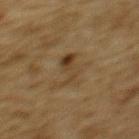Impression: Captured during whole-body skin photography for melanoma surveillance; the lesion was not biopsied. Context: The subject is a male aged approximately 85 or older. A 15 mm close-up extracted from a 3D total-body photography capture. Automated image analysis of the tile measured an average lesion color of about L≈34 a*≈12 b*≈28 (CIELAB), roughly 7 lightness units darker than nearby skin, and a lesion-to-skin contrast of about 6.5 (normalized; higher = more distinct). The lesion is on the upper back. Approximately 4 mm at its widest.